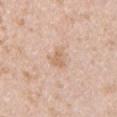Recorded during total-body skin imaging; not selected for excision or biopsy.